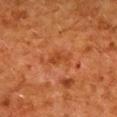workup: imaged on a skin check; not biopsied
patient: female, about 50 years old
automated lesion analysis: a lesion area of about 3.5 mm² and a shape-asymmetry score of about 0.35 (0 = symmetric); a mean CIELAB color near L≈37 a*≈26 b*≈35, roughly 6 lightness units darker than nearby skin, and a lesion-to-skin contrast of about 6 (normalized; higher = more distinct); a border-irregularity index near 3.5/10, a within-lesion color-variation index near 1/10, and a peripheral color-asymmetry measure near 0.5; a classifier nevus-likeness of about 0/100 and a detector confidence of about 100 out of 100 that the crop contains a lesion
lesion size: ≈3 mm
body site: the back
lighting: cross-polarized illumination
imaging modality: ~15 mm tile from a whole-body skin photo